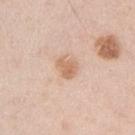biopsy_status: not biopsied; imaged during a skin examination
image:
  source: total-body photography crop
  field_of_view_mm: 15
lesion_size:
  long_diameter_mm_approx: 2.5
site: left upper arm
patient:
  sex: male
  age_approx: 40